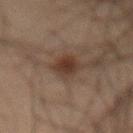Q: Was this lesion biopsied?
A: catalogued during a skin exam; not biopsied
Q: What are the patient's age and sex?
A: male, approximately 60 years of age
Q: How was this image acquired?
A: ~15 mm crop, total-body skin-cancer survey
Q: Lesion location?
A: the abdomen
Q: Automated lesion metrics?
A: internal color variation of about 2.5 on a 0–10 scale